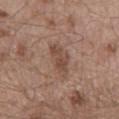image source: 15 mm crop, total-body photography | patient: male, roughly 55 years of age | anatomic site: the lower back | lesion size: ≈5 mm.